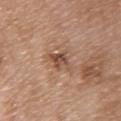Context:
An algorithmic analysis of the crop reported a classifier nevus-likeness of about 0/100. On the upper back. The tile uses white-light illumination. Measured at roughly 3.5 mm in maximum diameter. The patient is a female aged approximately 70. A 15 mm close-up tile from a total-body photography series done for melanoma screening.